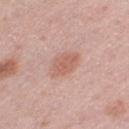No biopsy was performed on this lesion — it was imaged during a full skin examination and was not determined to be concerning. Located on the left thigh. Measured at roughly 3.5 mm in maximum diameter. A 15 mm close-up tile from a total-body photography series done for melanoma screening. A female subject in their 40s.